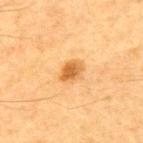Impression: The lesion was photographed on a routine skin check and not biopsied; there is no pathology result. Image and clinical context: A male subject, aged 63 to 67. About 2.5 mm across. A lesion tile, about 15 mm wide, cut from a 3D total-body photograph. On the upper back. Automated image analysis of the tile measured an area of roughly 4.5 mm², an outline eccentricity of about 0.65 (0 = round, 1 = elongated), and two-axis asymmetry of about 0.15. The software also gave a lesion color around L≈53 a*≈22 b*≈42 in CIELAB, about 11 CIELAB-L* units darker than the surrounding skin, and a lesion-to-skin contrast of about 8 (normalized; higher = more distinct). The tile uses cross-polarized illumination.